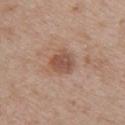{"biopsy_status": "not biopsied; imaged during a skin examination", "site": "upper back", "automated_metrics": {"cielab_L": 51, "cielab_a": 20, "cielab_b": 28, "vs_skin_darker_L": 10.0, "vs_skin_contrast_norm": 7.5, "color_variation_0_10": 2.5, "peripheral_color_asymmetry": 1.0}, "lighting": "white-light", "lesion_size": {"long_diameter_mm_approx": 3.0}, "patient": {"sex": "female", "age_approx": 50}, "image": {"source": "total-body photography crop", "field_of_view_mm": 15}}